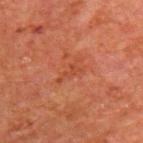<case>
<biopsy_status>not biopsied; imaged during a skin examination</biopsy_status>
<lighting>cross-polarized</lighting>
<site>upper back</site>
<image>
  <source>total-body photography crop</source>
  <field_of_view_mm>15</field_of_view_mm>
</image>
<patient>
  <sex>male</sex>
  <age_approx>65</age_approx>
</patient>
</case>